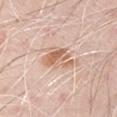Background:
From the arm. The lesion's longest dimension is about 4 mm. A male subject, in their 70s. A region of skin cropped from a whole-body photographic capture, roughly 15 mm wide. Imaged with white-light lighting. The lesion-visualizer software estimated an area of roughly 7 mm², a shape eccentricity near 0.75, and two-axis asymmetry of about 0.4. The software also gave a mean CIELAB color near L≈64 a*≈20 b*≈31. It also reported an automated nevus-likeness rating near 50 out of 100 and a detector confidence of about 100 out of 100 that the crop contains a lesion.